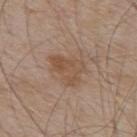patient — male, approximately 55 years of age
anatomic site — the upper back
image source — 15 mm crop, total-body photography
tile lighting — white-light illumination